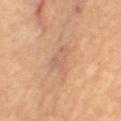follow-up: catalogued during a skin exam; not biopsied
lighting: cross-polarized
site: the left leg
image source: 15 mm crop, total-body photography
size: about 4 mm
patient: female, about 65 years old
automated lesion analysis: an outline eccentricity of about 0.75 (0 = round, 1 = elongated); an average lesion color of about L≈58 a*≈20 b*≈29 (CIELAB), about 6 CIELAB-L* units darker than the surrounding skin, and a lesion-to-skin contrast of about 5 (normalized; higher = more distinct)